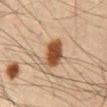<record>
  <biopsy_status>not biopsied; imaged during a skin examination</biopsy_status>
  <image>
    <source>total-body photography crop</source>
    <field_of_view_mm>15</field_of_view_mm>
  </image>
  <patient>
    <sex>male</sex>
    <age_approx>60</age_approx>
  </patient>
  <lighting>cross-polarized</lighting>
  <automated_metrics>
    <area_mm2_approx>8.5</area_mm2_approx>
    <nevus_likeness_0_100>100</nevus_likeness_0_100>
    <lesion_detection_confidence_0_100>100</lesion_detection_confidence_0_100>
  </automated_metrics>
  <site>abdomen</site>
  <lesion_size>
    <long_diameter_mm_approx>4.0</long_diameter_mm_approx>
  </lesion_size>
</record>